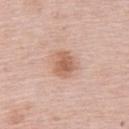Impression: The lesion was tiled from a total-body skin photograph and was not biopsied. Background: The subject is a female aged 63 to 67. Imaged with white-light lighting. Located on the upper back. A region of skin cropped from a whole-body photographic capture, roughly 15 mm wide. The lesion's longest dimension is about 3 mm.